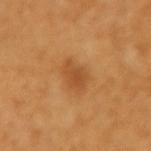Clinical impression: Recorded during total-body skin imaging; not selected for excision or biopsy. Context: The patient is a female aged around 55. From the left forearm. Measured at roughly 2.5 mm in maximum diameter. A region of skin cropped from a whole-body photographic capture, roughly 15 mm wide. Captured under cross-polarized illumination. The lesion-visualizer software estimated a shape eccentricity near 0.65 and a shape-asymmetry score of about 0.25 (0 = symmetric). It also reported a lesion color around L≈50 a*≈26 b*≈43 in CIELAB and a normalized lesion–skin contrast near 6.5. It also reported a border-irregularity index near 2.5/10 and a within-lesion color-variation index near 2/10. And it measured lesion-presence confidence of about 100/100.